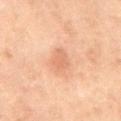The lesion was tiled from a total-body skin photograph and was not biopsied. A roughly 15 mm field-of-view crop from a total-body skin photograph. The tile uses cross-polarized illumination. On the abdomen. Measured at roughly 3 mm in maximum diameter. The lesion-visualizer software estimated a mean CIELAB color near L≈51 a*≈19 b*≈28, about 6 CIELAB-L* units darker than the surrounding skin, and a lesion-to-skin contrast of about 5 (normalized; higher = more distinct). The analysis additionally found a detector confidence of about 100 out of 100 that the crop contains a lesion. A male subject, approximately 70 years of age.